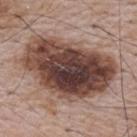Recorded during total-body skin imaging; not selected for excision or biopsy.
A roughly 15 mm field-of-view crop from a total-body skin photograph.
The subject is a male aged 63 to 67.
Located on the upper back.